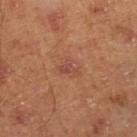This lesion was catalogued during total-body skin photography and was not selected for biopsy. Located on the right lower leg. Cropped from a whole-body photographic skin survey; the tile spans about 15 mm. A male patient, roughly 45 years of age.